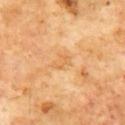biopsy status: catalogued during a skin exam; not biopsied
diameter: ~2.5 mm (longest diameter)
acquisition: ~15 mm crop, total-body skin-cancer survey
lighting: cross-polarized
subject: male, aged approximately 60
site: the front of the torso